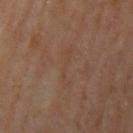Assessment:
The lesion was photographed on a routine skin check and not biopsied; there is no pathology result.
Background:
Measured at roughly 4.5 mm in maximum diameter. Located on the left upper arm. A 15 mm close-up tile from a total-body photography series done for melanoma screening. Automated tile analysis of the lesion measured an area of roughly 4 mm², a shape eccentricity near 0.95, and two-axis asymmetry of about 0.6. It also reported an average lesion color of about L≈41 a*≈17 b*≈26 (CIELAB), about 3 CIELAB-L* units darker than the surrounding skin, and a normalized lesion–skin contrast near 3. The software also gave a border-irregularity index near 8/10, a within-lesion color-variation index near 0/10, and radial color variation of about 0. The patient is a male about 60 years old.